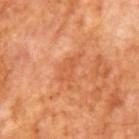Case summary:
* biopsy status · no biopsy performed (imaged during a skin exam)
* patient · male, about 65 years old
* acquisition · 15 mm crop, total-body photography
* lesion diameter · about 4.5 mm
* tile lighting · cross-polarized illumination
* automated lesion analysis · a footprint of about 6 mm², an eccentricity of roughly 0.9, and two-axis asymmetry of about 0.45; a lesion–skin lightness drop of about 6 and a lesion-to-skin contrast of about 4.5 (normalized; higher = more distinct)